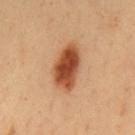workup: total-body-photography surveillance lesion; no biopsy
acquisition: ~15 mm tile from a whole-body skin photo
patient: male, approximately 50 years of age
location: the mid back
tile lighting: cross-polarized illumination
lesion diameter: about 6 mm
TBP lesion metrics: a lesion area of about 14 mm² and a shape-asymmetry score of about 0.2 (0 = symmetric); a mean CIELAB color near L≈50 a*≈26 b*≈37, roughly 17 lightness units darker than nearby skin, and a normalized lesion–skin contrast near 11.5; a border-irregularity rating of about 2.5/10, a within-lesion color-variation index near 6/10, and radial color variation of about 1.5; a classifier nevus-likeness of about 100/100 and lesion-presence confidence of about 100/100